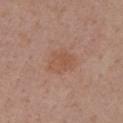subject: female, aged around 55
anatomic site: the right upper arm
TBP lesion metrics: a mean CIELAB color near L≈52 a*≈21 b*≈30, roughly 6 lightness units darker than nearby skin, and a normalized lesion–skin contrast near 5.5; a border-irregularity rating of about 3/10, a color-variation rating of about 2.5/10, and peripheral color asymmetry of about 0.5
image source: ~15 mm crop, total-body skin-cancer survey
diameter: ≈3.5 mm
tile lighting: white-light